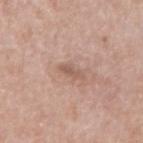Notes:
- biopsy status · catalogued during a skin exam; not biopsied
- image · ~15 mm crop, total-body skin-cancer survey
- subject · male, aged 73–77
- location · the right upper arm
- lesion diameter · about 3 mm
- automated lesion analysis · a footprint of about 3 mm², a shape eccentricity near 0.9, and a shape-asymmetry score of about 0.35 (0 = symmetric); a mean CIELAB color near L≈57 a*≈18 b*≈26 and a normalized lesion–skin contrast near 6; a border-irregularity rating of about 3.5/10, a color-variation rating of about 0.5/10, and peripheral color asymmetry of about 0; a nevus-likeness score of about 0/100